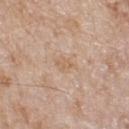{
  "biopsy_status": "not biopsied; imaged during a skin examination"
}